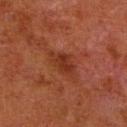A male patient aged 78 to 82. This is a cross-polarized tile. The lesion is on the leg. Cropped from a total-body skin-imaging series; the visible field is about 15 mm. The recorded lesion diameter is about 4 mm.